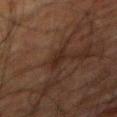Part of a total-body skin-imaging series; this lesion was reviewed on a skin check and was not flagged for biopsy. A 15 mm close-up extracted from a 3D total-body photography capture. A male subject, aged 78 to 82. From the abdomen.